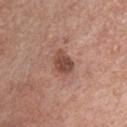The lesion was tiled from a total-body skin photograph and was not biopsied.
A male patient aged 63–67.
The lesion is located on the front of the torso.
A region of skin cropped from a whole-body photographic capture, roughly 15 mm wide.